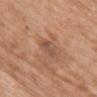Impression:
Imaged during a routine full-body skin examination; the lesion was not biopsied and no histopathology is available.
Acquisition and patient details:
Captured under white-light illumination. A female subject in their mid-60s. An algorithmic analysis of the crop reported a shape eccentricity near 0.8 and a shape-asymmetry score of about 0.4 (0 = symmetric). The analysis additionally found a mean CIELAB color near L≈51 a*≈20 b*≈29, a lesion–skin lightness drop of about 8, and a normalized border contrast of about 6. The analysis additionally found border irregularity of about 4 on a 0–10 scale and radial color variation of about 1. The software also gave an automated nevus-likeness rating near 0 out of 100 and lesion-presence confidence of about 100/100. This image is a 15 mm lesion crop taken from a total-body photograph. The lesion is on the back. Approximately 3 mm at its widest.